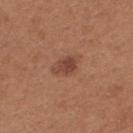The lesion was photographed on a routine skin check and not biopsied; there is no pathology result.
From the upper back.
Cropped from a total-body skin-imaging series; the visible field is about 15 mm.
The patient is a female aged 28–32.